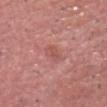The lesion was photographed on a routine skin check and not biopsied; there is no pathology result.
Captured under white-light illumination.
A 15 mm crop from a total-body photograph taken for skin-cancer surveillance.
The lesion is located on the head or neck.
The lesion's longest dimension is about 3 mm.
An algorithmic analysis of the crop reported a lesion area of about 4 mm².
A male patient aged approximately 75.